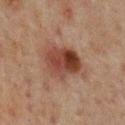follow-up: no biopsy performed (imaged during a skin exam)
location: the mid back
image source: ~15 mm tile from a whole-body skin photo
subject: male, aged 63 to 67
tile lighting: cross-polarized illumination